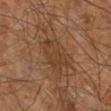biopsy_status: not biopsied; imaged during a skin examination
patient:
  sex: male
  age_approx: 60
site: right leg
image:
  source: total-body photography crop
  field_of_view_mm: 15